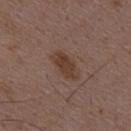Part of a total-body skin-imaging series; this lesion was reviewed on a skin check and was not flagged for biopsy.
From the mid back.
A male subject, in their 50s.
A 15 mm close-up extracted from a 3D total-body photography capture.
This is a white-light tile.
Measured at roughly 4 mm in maximum diameter.